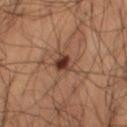{"biopsy_status": "not biopsied; imaged during a skin examination", "automated_metrics": {"area_mm2_approx": 4.5, "eccentricity": 0.35, "shape_asymmetry": 0.25, "cielab_L": 29, "cielab_a": 16, "cielab_b": 22, "vs_skin_darker_L": 10.0, "vs_skin_contrast_norm": 9.5, "border_irregularity_0_10": 2.0, "color_variation_0_10": 5.5}, "site": "right thigh", "lighting": "cross-polarized", "patient": {"sex": "male", "age_approx": 50}, "image": {"source": "total-body photography crop", "field_of_view_mm": 15}}